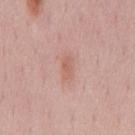{
  "biopsy_status": "not biopsied; imaged during a skin examination",
  "lesion_size": {
    "long_diameter_mm_approx": 2.5
  },
  "image": {
    "source": "total-body photography crop",
    "field_of_view_mm": 15
  },
  "automated_metrics": {
    "vs_skin_darker_L": 7.0,
    "vs_skin_contrast_norm": 5.5,
    "peripheral_color_asymmetry": 0.0
  },
  "patient": {
    "sex": "male",
    "age_approx": 60
  },
  "site": "chest"
}